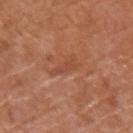Recorded during total-body skin imaging; not selected for excision or biopsy. About 4 mm across. The patient is a female in their mid- to late 60s. A roughly 15 mm field-of-view crop from a total-body skin photograph. From the left arm. The total-body-photography lesion software estimated an average lesion color of about L≈48 a*≈25 b*≈33 (CIELAB), about 6 CIELAB-L* units darker than the surrounding skin, and a normalized lesion–skin contrast near 4.5.